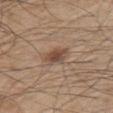{
  "biopsy_status": "not biopsied; imaged during a skin examination",
  "patient": {
    "sex": "male",
    "age_approx": 50
  },
  "site": "upper back",
  "image": {
    "source": "total-body photography crop",
    "field_of_view_mm": 15
  },
  "lighting": "white-light"
}